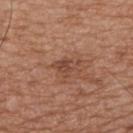| field | value |
|---|---|
| notes | no biopsy performed (imaged during a skin exam) |
| subject | male, approximately 65 years of age |
| anatomic site | the upper back |
| lesion size | ~3.5 mm (longest diameter) |
| image source | ~15 mm crop, total-body skin-cancer survey |
| tile lighting | white-light illumination |